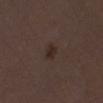{
  "site": "right lower leg",
  "lesion_size": {
    "long_diameter_mm_approx": 2.5
  },
  "image": {
    "source": "total-body photography crop",
    "field_of_view_mm": 15
  },
  "patient": {
    "sex": "female",
    "age_approx": 50
  }
}